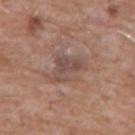biopsy_status: not biopsied; imaged during a skin examination
lesion_size:
  long_diameter_mm_approx: 4.0
patient:
  sex: male
  age_approx: 60
site: chest
lighting: white-light
image:
  source: total-body photography crop
  field_of_view_mm: 15
automated_metrics:
  nevus_likeness_0_100: 0
  lesion_detection_confidence_0_100: 100